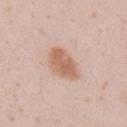Q: How large is the lesion?
A: ≈4.5 mm
Q: Patient demographics?
A: male, approximately 40 years of age
Q: Lesion location?
A: the right upper arm
Q: What did automated image analysis measure?
A: a footprint of about 9.5 mm², a shape eccentricity near 0.8, and a symmetry-axis asymmetry near 0.2; an average lesion color of about L≈62 a*≈20 b*≈30 (CIELAB) and about 11 CIELAB-L* units darker than the surrounding skin; a nevus-likeness score of about 90/100 and lesion-presence confidence of about 100/100
Q: Illumination type?
A: white-light illumination
Q: How was this image acquired?
A: ~15 mm crop, total-body skin-cancer survey